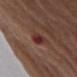Clinical impression: The lesion was tiled from a total-body skin photograph and was not biopsied. Background: The recorded lesion diameter is about 3.5 mm. The patient is a male about 75 years old. Located on the right upper arm. The tile uses white-light illumination. The total-body-photography lesion software estimated internal color variation of about 3 on a 0–10 scale and a peripheral color-asymmetry measure near 1. A lesion tile, about 15 mm wide, cut from a 3D total-body photograph.